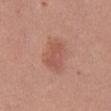Automated image analysis of the tile measured an area of roughly 7.5 mm², a shape eccentricity near 0.75, and a shape-asymmetry score of about 0.25 (0 = symmetric). And it measured a lesion color around L≈54 a*≈25 b*≈28 in CIELAB, about 8 CIELAB-L* units darker than the surrounding skin, and a lesion-to-skin contrast of about 5.5 (normalized; higher = more distinct). The analysis additionally found a border-irregularity rating of about 3/10 and peripheral color asymmetry of about 1. The software also gave a detector confidence of about 100 out of 100 that the crop contains a lesion. A female patient, in their mid-30s. A lesion tile, about 15 mm wide, cut from a 3D total-body photograph. Captured under white-light illumination. Located on the abdomen.